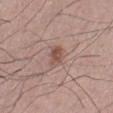Imaged during a routine full-body skin examination; the lesion was not biopsied and no histopathology is available. A male subject aged 53–57. A 15 mm close-up tile from a total-body photography series done for melanoma screening.